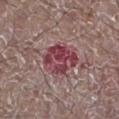follow-up: total-body-photography surveillance lesion; no biopsy
lighting: white-light illumination
subject: male, in their mid- to late 60s
location: the leg
image: total-body-photography crop, ~15 mm field of view
diameter: about 5 mm